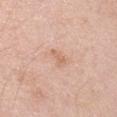Assessment: The lesion was photographed on a routine skin check and not biopsied; there is no pathology result. Context: From the right upper arm. This is a white-light tile. The lesion's longest dimension is about 2.5 mm. A male patient, roughly 45 years of age. Cropped from a whole-body photographic skin survey; the tile spans about 15 mm.